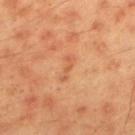<tbp_lesion>
<biopsy_status>not biopsied; imaged during a skin examination</biopsy_status>
<automated_metrics>
  <area_mm2_approx>3.0</area_mm2_approx>
  <eccentricity>0.95</eccentricity>
  <shape_asymmetry>0.45</shape_asymmetry>
  <vs_skin_darker_L>7.0</vs_skin_darker_L>
  <vs_skin_contrast_norm>5.0</vs_skin_contrast_norm>
  <border_irregularity_0_10>5.5</border_irregularity_0_10>
  <color_variation_0_10>0.0</color_variation_0_10>
  <peripheral_color_asymmetry>0.0</peripheral_color_asymmetry>
  <nevus_likeness_0_100>0</nevus_likeness_0_100>
  <lesion_detection_confidence_0_100>100</lesion_detection_confidence_0_100>
</automated_metrics>
<patient>
  <sex>male</sex>
  <age_approx>45</age_approx>
</patient>
<lighting>cross-polarized</lighting>
<image>
  <source>total-body photography crop</source>
  <field_of_view_mm>15</field_of_view_mm>
</image>
<lesion_size>
  <long_diameter_mm_approx>3.0</long_diameter_mm_approx>
</lesion_size>
<site>upper back</site>
</tbp_lesion>